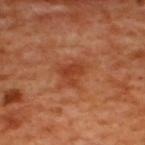Findings:
• body site: the upper back
• size: ~3 mm (longest diameter)
• illumination: cross-polarized illumination
• image-analysis metrics: a lesion area of about 5.5 mm² and a shape-asymmetry score of about 0.4 (0 = symmetric); a lesion–skin lightness drop of about 8 and a lesion-to-skin contrast of about 6.5 (normalized; higher = more distinct); a lesion-detection confidence of about 100/100
• patient: male, aged around 50
• image: ~15 mm tile from a whole-body skin photo